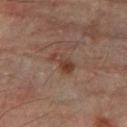Clinical summary: A male subject, aged 43 to 47. Measured at roughly 3.5 mm in maximum diameter. The lesion is on the arm. Automated image analysis of the tile measured a lesion color around L≈33 a*≈17 b*≈22 in CIELAB, a lesion–skin lightness drop of about 7, and a normalized border contrast of about 7.5. The software also gave border irregularity of about 4 on a 0–10 scale, a within-lesion color-variation index near 3.5/10, and radial color variation of about 1. The software also gave a nevus-likeness score of about 15/100. Cropped from a total-body skin-imaging series; the visible field is about 15 mm.